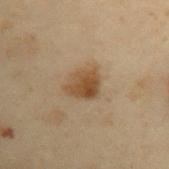workup — no biopsy performed (imaged during a skin exam) | acquisition — 15 mm crop, total-body photography | patient — male, in their mid- to late 50s | TBP lesion metrics — a mean CIELAB color near L≈39 a*≈13 b*≈29, roughly 9 lightness units darker than nearby skin, and a lesion-to-skin contrast of about 8.5 (normalized; higher = more distinct); a border-irregularity rating of about 2.5/10, a color-variation rating of about 4/10, and a peripheral color-asymmetry measure near 1.5; a lesion-detection confidence of about 100/100 | lesion size — about 3.5 mm | illumination — cross-polarized illumination | site — the left upper arm.